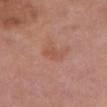No biopsy was performed on this lesion — it was imaged during a full skin examination and was not determined to be concerning. Cropped from a whole-body photographic skin survey; the tile spans about 15 mm. On the chest. A female subject, aged 63 to 67. An algorithmic analysis of the crop reported a lesion area of about 5 mm², an outline eccentricity of about 0.85 (0 = round, 1 = elongated), and a symmetry-axis asymmetry near 0.35. The analysis additionally found border irregularity of about 3.5 on a 0–10 scale and a within-lesion color-variation index near 1.5/10. It also reported a nevus-likeness score of about 0/100. Captured under white-light illumination.